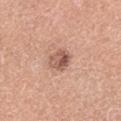Recorded during total-body skin imaging; not selected for excision or biopsy.
The recorded lesion diameter is about 3 mm.
A roughly 15 mm field-of-view crop from a total-body skin photograph.
On the left lower leg.
A female patient about 50 years old.
Automated image analysis of the tile measured a mean CIELAB color near L≈56 a*≈22 b*≈27 and about 12 CIELAB-L* units darker than the surrounding skin. The analysis additionally found a border-irregularity index near 2/10, a within-lesion color-variation index near 8/10, and a peripheral color-asymmetry measure near 3.5. The analysis additionally found a nevus-likeness score of about 15/100 and a detector confidence of about 100 out of 100 that the crop contains a lesion.
The tile uses white-light illumination.